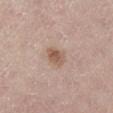This lesion was catalogued during total-body skin photography and was not selected for biopsy. This is a white-light tile. The lesion-visualizer software estimated an outline eccentricity of about 0.6 (0 = round, 1 = elongated). It also reported an average lesion color of about L≈56 a*≈18 b*≈27 (CIELAB), a lesion–skin lightness drop of about 10, and a normalized border contrast of about 7.5. And it measured a border-irregularity index near 2/10 and internal color variation of about 3 on a 0–10 scale. A male subject aged around 70. The lesion is located on the right lower leg. A 15 mm crop from a total-body photograph taken for skin-cancer surveillance. The lesion's longest dimension is about 2.5 mm.